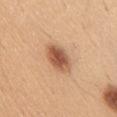No biopsy was performed on this lesion — it was imaged during a full skin examination and was not determined to be concerning.
Approximately 4.5 mm at its widest.
A male patient, approximately 50 years of age.
The lesion is located on the front of the torso.
A 15 mm close-up extracted from a 3D total-body photography capture.